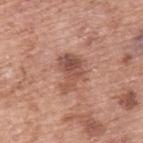Clinical impression:
Imaged during a routine full-body skin examination; the lesion was not biopsied and no histopathology is available.
Context:
The subject is a male aged approximately 55. Cropped from a total-body skin-imaging series; the visible field is about 15 mm. Imaged with white-light lighting. Measured at roughly 4.5 mm in maximum diameter. The lesion is on the upper back.